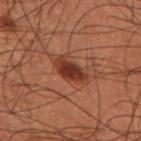Recorded during total-body skin imaging; not selected for excision or biopsy. The tile uses cross-polarized illumination. The lesion's longest dimension is about 3.5 mm. A close-up tile cropped from a whole-body skin photograph, about 15 mm across. A male patient in their 60s. On the left forearm.